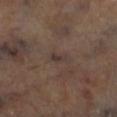Located on the right lower leg.
This image is a 15 mm lesion crop taken from a total-body photograph.
This is a cross-polarized tile.